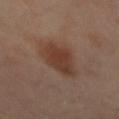biopsy_status: not biopsied; imaged during a skin examination
lighting: cross-polarized
site: mid back
automated_metrics:
  cielab_L: 39
  cielab_a: 20
  cielab_b: 27
  vs_skin_darker_L: 9.0
  vs_skin_contrast_norm: 8.0
image:
  source: total-body photography crop
  field_of_view_mm: 15
lesion_size:
  long_diameter_mm_approx: 5.5